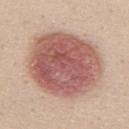Impression: Part of a total-body skin-imaging series; this lesion was reviewed on a skin check and was not flagged for biopsy. Image and clinical context: The lesion is on the front of the torso. A 15 mm crop from a total-body photograph taken for skin-cancer surveillance. An algorithmic analysis of the crop reported a lesion area of about 55 mm² and an outline eccentricity of about 0.3 (0 = round, 1 = elongated). The analysis additionally found an automated nevus-likeness rating near 100 out of 100 and a detector confidence of about 100 out of 100 that the crop contains a lesion. This is a white-light tile. A female patient, aged approximately 50.